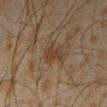notes: no biopsy performed (imaged during a skin exam) | location: the left forearm | imaging modality: ~15 mm tile from a whole-body skin photo | image-analysis metrics: a symmetry-axis asymmetry near 0.3; border irregularity of about 3 on a 0–10 scale and a within-lesion color-variation index near 1.5/10 | patient: male, in their mid- to late 40s | illumination: cross-polarized.